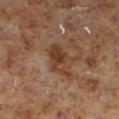The lesion was photographed on a routine skin check and not biopsied; there is no pathology result.
The lesion is located on the left lower leg.
Cropped from a total-body skin-imaging series; the visible field is about 15 mm.
The subject is a male aged approximately 60.